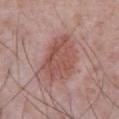Imaged during a routine full-body skin examination; the lesion was not biopsied and no histopathology is available. About 6.5 mm across. On the front of the torso. An algorithmic analysis of the crop reported about 9 CIELAB-L* units darker than the surrounding skin and a normalized lesion–skin contrast near 7. And it measured a border-irregularity rating of about 2.5/10, a color-variation rating of about 3.5/10, and a peripheral color-asymmetry measure near 1. Cropped from a whole-body photographic skin survey; the tile spans about 15 mm. A male subject roughly 65 years of age. This is a white-light tile.